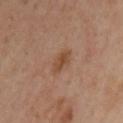From the left upper arm.
This image is a 15 mm lesion crop taken from a total-body photograph.
A female subject, aged 38 to 42.
The tile uses cross-polarized illumination.
About 3 mm across.